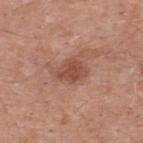<case>
  <biopsy_status>not biopsied; imaged during a skin examination</biopsy_status>
  <automated_metrics>
    <vs_skin_darker_L>10.0</vs_skin_darker_L>
    <nevus_likeness_0_100>30</nevus_likeness_0_100>
    <lesion_detection_confidence_0_100>100</lesion_detection_confidence_0_100>
  </automated_metrics>
  <patient>
    <sex>male</sex>
    <age_approx>55</age_approx>
  </patient>
  <image>
    <source>total-body photography crop</source>
    <field_of_view_mm>15</field_of_view_mm>
  </image>
  <lesion_size>
    <long_diameter_mm_approx>4.0</long_diameter_mm_approx>
  </lesion_size>
  <site>upper back</site>
  <lighting>white-light</lighting>
</case>